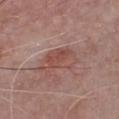Imaged during a routine full-body skin examination; the lesion was not biopsied and no histopathology is available. The lesion's longest dimension is about 5 mm. A male patient, approximately 65 years of age. The total-body-photography lesion software estimated a lesion color around L≈49 a*≈23 b*≈24 in CIELAB and a lesion–skin lightness drop of about 8. It also reported a nevus-likeness score of about 5/100 and a lesion-detection confidence of about 100/100. Cropped from a total-body skin-imaging series; the visible field is about 15 mm. The lesion is located on the chest.